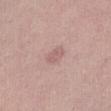- biopsy status: total-body-photography surveillance lesion; no biopsy
- lighting: white-light illumination
- subject: female, aged 38–42
- automated metrics: a mean CIELAB color near L≈60 a*≈21 b*≈21, about 7 CIELAB-L* units darker than the surrounding skin, and a normalized lesion–skin contrast near 5; a border-irregularity rating of about 1.5/10, a within-lesion color-variation index near 1.5/10, and radial color variation of about 0.5
- diameter: ≈2.5 mm
- image: total-body-photography crop, ~15 mm field of view
- location: the abdomen